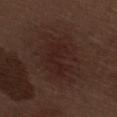{
  "biopsy_status": "not biopsied; imaged during a skin examination",
  "lighting": "white-light",
  "lesion_size": {
    "long_diameter_mm_approx": 7.5
  },
  "site": "right thigh",
  "automated_metrics": {
    "area_mm2_approx": 29.0,
    "eccentricity": 0.6,
    "shape_asymmetry": 0.25,
    "cielab_L": 23,
    "cielab_a": 16,
    "cielab_b": 20,
    "vs_skin_darker_L": 4.0,
    "vs_skin_contrast_norm": 5.5,
    "nevus_likeness_0_100": 15,
    "lesion_detection_confidence_0_100": 100
  },
  "image": {
    "source": "total-body photography crop",
    "field_of_view_mm": 15
  },
  "patient": {
    "sex": "male",
    "age_approx": 70
  }
}